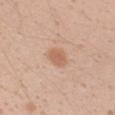{
  "biopsy_status": "not biopsied; imaged during a skin examination",
  "lighting": "white-light",
  "patient": {
    "sex": "female",
    "age_approx": 20
  },
  "site": "right forearm",
  "automated_metrics": {
    "border_irregularity_0_10": 1.5,
    "peripheral_color_asymmetry": 1.0,
    "nevus_likeness_0_100": 75,
    "lesion_detection_confidence_0_100": 100
  },
  "image": {
    "source": "total-body photography crop",
    "field_of_view_mm": 15
  }
}